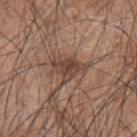| feature | finding |
|---|---|
| biopsy status | catalogued during a skin exam; not biopsied |
| subject | male, roughly 45 years of age |
| imaging modality | ~15 mm crop, total-body skin-cancer survey |
| tile lighting | white-light |
| anatomic site | the upper back |
| diameter | ~4 mm (longest diameter) |